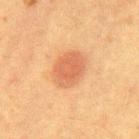The lesion was tiled from a total-body skin photograph and was not biopsied. On the mid back. This is a cross-polarized tile. The subject is a female in their 40s. Cropped from a total-body skin-imaging series; the visible field is about 15 mm. Automated tile analysis of the lesion measured an eccentricity of roughly 0.7 and a shape-asymmetry score of about 0.1 (0 = symmetric). The analysis additionally found a mean CIELAB color near L≈53 a*≈24 b*≈35, a lesion–skin lightness drop of about 9, and a normalized border contrast of about 6.5. It also reported a border-irregularity index near 1/10. The lesion's longest dimension is about 4.5 mm.